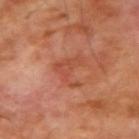location = the right upper arm; acquisition = ~15 mm tile from a whole-body skin photo; lighting = cross-polarized; subject = male, aged 68 to 72.